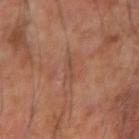The lesion was tiled from a total-body skin photograph and was not biopsied. From the arm. A close-up tile cropped from a whole-body skin photograph, about 15 mm across. A male patient, about 60 years old. The lesion's longest dimension is about 3 mm.